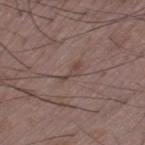Q: How was this image acquired?
A: 15 mm crop, total-body photography
Q: How large is the lesion?
A: ≈3 mm
Q: What did automated image analysis measure?
A: a mean CIELAB color near L≈44 a*≈16 b*≈21, a lesion–skin lightness drop of about 7, and a lesion-to-skin contrast of about 6 (normalized; higher = more distinct); a border-irregularity rating of about 7/10, internal color variation of about 0 on a 0–10 scale, and peripheral color asymmetry of about 0
Q: Lesion location?
A: the left thigh
Q: Who is the patient?
A: male, aged 48 to 52
Q: What lighting was used for the tile?
A: white-light